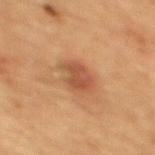{"biopsy_status": "not biopsied; imaged during a skin examination", "lesion_size": {"long_diameter_mm_approx": 3.5}, "site": "upper back", "lighting": "cross-polarized", "patient": {"sex": "female", "age_approx": 70}, "automated_metrics": {"shape_asymmetry": 0.2, "vs_skin_darker_L": 8.0, "vs_skin_contrast_norm": 7.0, "color_variation_0_10": 2.5, "peripheral_color_asymmetry": 1.0, "lesion_detection_confidence_0_100": 100}, "image": {"source": "total-body photography crop", "field_of_view_mm": 15}}